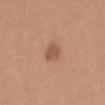  biopsy_status: not biopsied; imaged during a skin examination
  patient:
    sex: female
    age_approx: 20
  lighting: white-light
  site: mid back
  automated_metrics:
    area_mm2_approx: 4.0
    shape_asymmetry: 0.25
    vs_skin_darker_L: 9.0
    vs_skin_contrast_norm: 6.5
    nevus_likeness_0_100: 50
  lesion_size:
    long_diameter_mm_approx: 2.5
  image:
    source: total-body photography crop
    field_of_view_mm: 15